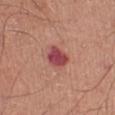{
  "biopsy_status": "not biopsied; imaged during a skin examination",
  "patient": {
    "sex": "male",
    "age_approx": 60
  },
  "image": {
    "source": "total-body photography crop",
    "field_of_view_mm": 15
  },
  "site": "left lower leg"
}